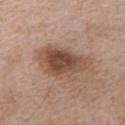Findings:
• subject — male, approximately 65 years of age
• acquisition — 15 mm crop, total-body photography
• site — the chest
• TBP lesion metrics — a lesion area of about 19 mm², an eccentricity of roughly 0.75, and a symmetry-axis asymmetry near 0.3; border irregularity of about 3.5 on a 0–10 scale and a peripheral color-asymmetry measure near 1.5; an automated nevus-likeness rating near 85 out of 100 and a detector confidence of about 100 out of 100 that the crop contains a lesion
• diameter — ~6 mm (longest diameter)
• illumination — white-light